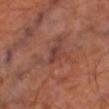Notes:
– follow-up — total-body-photography surveillance lesion; no biopsy
– image source — ~15 mm tile from a whole-body skin photo
– location — the right thigh
– patient — male, about 65 years old